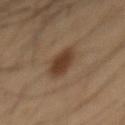Part of a total-body skin-imaging series; this lesion was reviewed on a skin check and was not flagged for biopsy. This is a cross-polarized tile. The subject is a male aged 43–47. This image is a 15 mm lesion crop taken from a total-body photograph. The recorded lesion diameter is about 4.5 mm. On the mid back.